From the mid back. The patient is a female aged 63–67. This image is a 15 mm lesion crop taken from a total-body photograph: 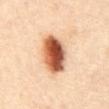Captured under cross-polarized illumination.
An algorithmic analysis of the crop reported a mean CIELAB color near L≈60 a*≈27 b*≈37 and a lesion-to-skin contrast of about 13.5 (normalized; higher = more distinct). It also reported a color-variation rating of about 10/10 and peripheral color asymmetry of about 4.
The lesion's longest dimension is about 6 mm.
On biopsy, histopathology showed an atypical melanocytic neoplasm (borderline).